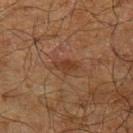<case>
  <biopsy_status>not biopsied; imaged during a skin examination</biopsy_status>
  <patient>
    <sex>male</sex>
    <age_approx>70</age_approx>
  </patient>
  <site>left upper arm</site>
  <lighting>cross-polarized</lighting>
  <lesion_size>
    <long_diameter_mm_approx>2.5</long_diameter_mm_approx>
  </lesion_size>
  <image>
    <source>total-body photography crop</source>
    <field_of_view_mm>15</field_of_view_mm>
  </image>
</case>